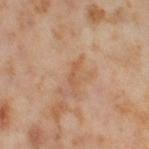workup = catalogued during a skin exam; not biopsied | size = about 3 mm | subject = female, aged approximately 55 | image = ~15 mm tile from a whole-body skin photo | location = the right thigh | tile lighting = cross-polarized.